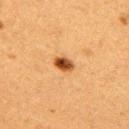Captured during whole-body skin photography for melanoma surveillance; the lesion was not biopsied. The lesion-visualizer software estimated a footprint of about 4.5 mm², a shape eccentricity near 0.7, and two-axis asymmetry of about 0.2. And it measured a mean CIELAB color near L≈44 a*≈23 b*≈38, about 16 CIELAB-L* units darker than the surrounding skin, and a lesion-to-skin contrast of about 11.5 (normalized; higher = more distinct). The software also gave internal color variation of about 6.5 on a 0–10 scale and radial color variation of about 2.5. The recorded lesion diameter is about 2.5 mm. A female patient aged 38–42. A close-up tile cropped from a whole-body skin photograph, about 15 mm across. The lesion is on the arm. Captured under cross-polarized illumination.